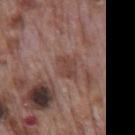| feature | finding |
|---|---|
| biopsy status | imaged on a skin check; not biopsied |
| location | the mid back |
| patient | male, roughly 70 years of age |
| imaging modality | total-body-photography crop, ~15 mm field of view |